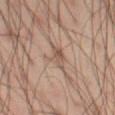notes — total-body-photography surveillance lesion; no biopsy
acquisition — total-body-photography crop, ~15 mm field of view
tile lighting — cross-polarized
site — the left thigh
lesion diameter — ≈3 mm
patient — male, aged 48 to 52